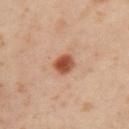Q: Was a biopsy performed?
A: total-body-photography surveillance lesion; no biopsy
Q: Lesion location?
A: the left upper arm
Q: What did automated image analysis measure?
A: a lesion color around L≈52 a*≈27 b*≈35 in CIELAB, roughly 16 lightness units darker than nearby skin, and a normalized lesion–skin contrast near 11; an automated nevus-likeness rating near 100 out of 100
Q: What is the imaging modality?
A: total-body-photography crop, ~15 mm field of view
Q: What is the lesion's diameter?
A: ~2.5 mm (longest diameter)
Q: What lighting was used for the tile?
A: cross-polarized
Q: Patient demographics?
A: female, aged 38–42